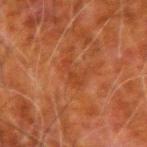Q: Was a biopsy performed?
A: no biopsy performed (imaged during a skin exam)
Q: How was this image acquired?
A: 15 mm crop, total-body photography
Q: What are the patient's age and sex?
A: male, aged around 80
Q: Lesion size?
A: ≈3 mm
Q: Lesion location?
A: the left upper arm
Q: How was the tile lit?
A: cross-polarized